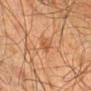The lesion was tiled from a total-body skin photograph and was not biopsied. Cropped from a total-body skin-imaging series; the visible field is about 15 mm. Longest diameter approximately 2.5 mm. Located on the left forearm. A male patient aged 78–82.